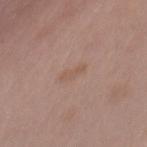This is a white-light tile. A 15 mm crop from a total-body photograph taken for skin-cancer surveillance. Automated tile analysis of the lesion measured an outline eccentricity of about 0.9 (0 = round, 1 = elongated). The analysis additionally found a border-irregularity index near 3/10, internal color variation of about 0 on a 0–10 scale, and a peripheral color-asymmetry measure near 0. And it measured an automated nevus-likeness rating near 0 out of 100 and a detector confidence of about 100 out of 100 that the crop contains a lesion. Approximately 2.5 mm at its widest. On the front of the torso. The patient is a female about 40 years old.